Automated tile analysis of the lesion measured an area of roughly 50 mm² and an outline eccentricity of about 0.55 (0 = round, 1 = elongated). It also reported a lesion–skin lightness drop of about 23. This is a white-light tile. Approximately 9 mm at its widest. Located on the mid back. A male patient aged 48 to 52. Cropped from a total-body skin-imaging series; the visible field is about 15 mm: 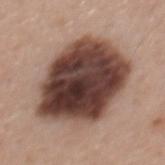Q: What did pathology find?
A: an invasive melanoma, superficial spreading type, Breslow thickness 0.4 mm and mitotic rate <1/mm²